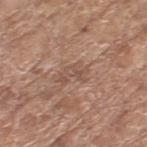Recorded during total-body skin imaging; not selected for excision or biopsy. This image is a 15 mm lesion crop taken from a total-body photograph. A female subject aged around 75. Longest diameter approximately 3 mm. The lesion is located on the left thigh.